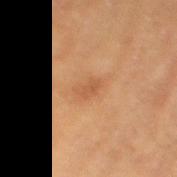workup: no biopsy performed (imaged during a skin exam)
image: ~15 mm tile from a whole-body skin photo
patient: male, aged approximately 85 or older
body site: the left thigh
TBP lesion metrics: an area of roughly 3 mm², an eccentricity of roughly 0.85, and a symmetry-axis asymmetry near 0.25; a border-irregularity index near 2.5/10 and peripheral color asymmetry of about 0.5; an automated nevus-likeness rating near 5 out of 100